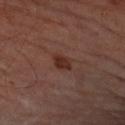The lesion was photographed on a routine skin check and not biopsied; there is no pathology result. A male patient roughly 65 years of age. A 15 mm close-up extracted from a 3D total-body photography capture. An algorithmic analysis of the crop reported a shape eccentricity near 0.75 and a shape-asymmetry score of about 0.2 (0 = symmetric). It also reported an average lesion color of about L≈28 a*≈20 b*≈23 (CIELAB). The software also gave a border-irregularity index near 2/10, a within-lesion color-variation index near 2/10, and peripheral color asymmetry of about 1. Located on the arm.